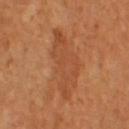Cropped from a whole-body photographic skin survey; the tile spans about 15 mm.
The patient is a female in their mid-50s.
Captured under cross-polarized illumination.
The lesion is located on the leg.
The lesion's longest dimension is about 8.5 mm.